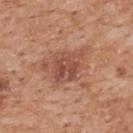Imaged during a routine full-body skin examination; the lesion was not biopsied and no histopathology is available. The lesion is on the back. A male patient, aged approximately 60. Automated image analysis of the tile measured a lesion color around L≈50 a*≈24 b*≈29 in CIELAB, roughly 9 lightness units darker than nearby skin, and a lesion-to-skin contrast of about 7 (normalized; higher = more distinct). The analysis additionally found a border-irregularity index near 3.5/10 and a within-lesion color-variation index near 5/10. It also reported a nevus-likeness score of about 5/100 and lesion-presence confidence of about 100/100. Longest diameter approximately 4 mm. A roughly 15 mm field-of-view crop from a total-body skin photograph.